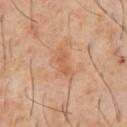lesion diameter: ≈3 mm
acquisition: ~15 mm crop, total-body skin-cancer survey
location: the chest
patient: male, roughly 60 years of age